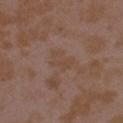The recorded lesion diameter is about 3 mm. Located on the left upper arm. Captured under white-light illumination. A female patient, about 35 years old. This image is a 15 mm lesion crop taken from a total-body photograph.